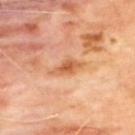| key | value |
|---|---|
| follow-up | total-body-photography surveillance lesion; no biopsy |
| body site | the upper back |
| imaging modality | ~15 mm crop, total-body skin-cancer survey |
| size | ≈3.5 mm |
| subject | male, approximately 65 years of age |